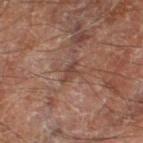Case summary:
* tile lighting: cross-polarized
* subject: male, aged 68 to 72
* image: ~15 mm crop, total-body skin-cancer survey
* site: the right thigh
* lesion diameter: ~3 mm (longest diameter)